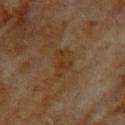Imaged during a routine full-body skin examination; the lesion was not biopsied and no histopathology is available. Automated tile analysis of the lesion measured an area of roughly 5.5 mm², an eccentricity of roughly 0.85, and two-axis asymmetry of about 0.4. It also reported a lesion color around L≈31 a*≈15 b*≈29 in CIELAB and a lesion–skin lightness drop of about 5. A female patient aged around 60. The lesion is on the upper back. A 15 mm crop from a total-body photograph taken for skin-cancer surveillance. Measured at roughly 3.5 mm in maximum diameter. The tile uses cross-polarized illumination.